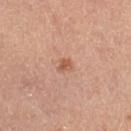This lesion was catalogued during total-body skin photography and was not selected for biopsy.
Approximately 2 mm at its widest.
A female subject, in their mid- to late 60s.
A region of skin cropped from a whole-body photographic capture, roughly 15 mm wide.
The tile uses cross-polarized illumination.
On the left thigh.